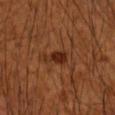Impression:
Part of a total-body skin-imaging series; this lesion was reviewed on a skin check and was not flagged for biopsy.
Image and clinical context:
On the right forearm. The patient is a male in their mid- to late 40s. Automated image analysis of the tile measured a symmetry-axis asymmetry near 0.35. It also reported an automated nevus-likeness rating near 45 out of 100 and a lesion-detection confidence of about 100/100. Longest diameter approximately 3.5 mm. A 15 mm crop from a total-body photograph taken for skin-cancer surveillance. Captured under cross-polarized illumination.